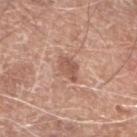{
  "biopsy_status": "not biopsied; imaged during a skin examination",
  "site": "left lower leg",
  "image": {
    "source": "total-body photography crop",
    "field_of_view_mm": 15
  },
  "automated_metrics": {
    "area_mm2_approx": 4.5,
    "eccentricity": 0.7,
    "shape_asymmetry": 0.3,
    "border_irregularity_0_10": 3.0,
    "color_variation_0_10": 2.0,
    "peripheral_color_asymmetry": 1.0,
    "nevus_likeness_0_100": 10
  },
  "patient": {
    "sex": "male",
    "age_approx": 80
  }
}